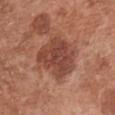biopsy status: total-body-photography surveillance lesion; no biopsy
lesion diameter: ≈5 mm
anatomic site: the chest
subject: female, approximately 65 years of age
image: ~15 mm crop, total-body skin-cancer survey